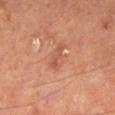Imaged during a routine full-body skin examination; the lesion was not biopsied and no histopathology is available.
The lesion-visualizer software estimated internal color variation of about 0 on a 0–10 scale and a peripheral color-asymmetry measure near 0. And it measured a classifier nevus-likeness of about 0/100 and a lesion-detection confidence of about 100/100.
Captured under cross-polarized illumination.
A male subject, in their mid-60s.
A lesion tile, about 15 mm wide, cut from a 3D total-body photograph.
The lesion is located on the right lower leg.
The lesion's longest dimension is about 3 mm.